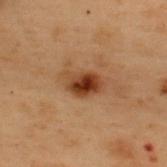* biopsy status — imaged on a skin check; not biopsied
* size — ~4.5 mm (longest diameter)
* image — ~15 mm tile from a whole-body skin photo
* patient — male, in their mid- to late 50s
* site — the upper back